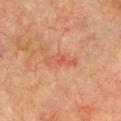follow-up = no biopsy performed (imaged during a skin exam)
patient = male, aged around 75
lesion diameter = ≈4.5 mm
lighting = cross-polarized illumination
anatomic site = the left upper arm
acquisition = ~15 mm tile from a whole-body skin photo